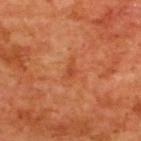  biopsy_status: not biopsied; imaged during a skin examination
  site: upper back
  patient:
    sex: male
    age_approx: 65
  automated_metrics:
    area_mm2_approx: 3.0
    eccentricity: 0.8
    shape_asymmetry: 0.5
    border_irregularity_0_10: 4.5
    color_variation_0_10: 1.0
    nevus_likeness_0_100: 0
    lesion_detection_confidence_0_100: 100
  image:
    source: total-body photography crop
    field_of_view_mm: 15
  lesion_size:
    long_diameter_mm_approx: 2.5